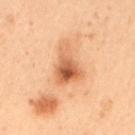| key | value |
|---|---|
| lighting | cross-polarized illumination |
| site | the mid back |
| lesion diameter | ~5 mm (longest diameter) |
| automated lesion analysis | internal color variation of about 8.5 on a 0–10 scale and radial color variation of about 3 |
| subject | female, aged 48–52 |
| image | ~15 mm tile from a whole-body skin photo |